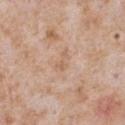This lesion was catalogued during total-body skin photography and was not selected for biopsy. Located on the front of the torso. A region of skin cropped from a whole-body photographic capture, roughly 15 mm wide. Approximately 3 mm at its widest. A male patient, approximately 65 years of age. An algorithmic analysis of the crop reported a lesion area of about 3 mm², an outline eccentricity of about 0.85 (0 = round, 1 = elongated), and a shape-asymmetry score of about 0.6 (0 = symmetric). And it measured a border-irregularity rating of about 6/10, internal color variation of about 0.5 on a 0–10 scale, and radial color variation of about 0. Captured under white-light illumination.